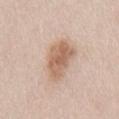Part of a total-body skin-imaging series; this lesion was reviewed on a skin check and was not flagged for biopsy.
The lesion-visualizer software estimated an outline eccentricity of about 0.65 (0 = round, 1 = elongated) and a symmetry-axis asymmetry near 0.25.
The patient is a female roughly 60 years of age.
This is a white-light tile.
Cropped from a whole-body photographic skin survey; the tile spans about 15 mm.
Located on the back.